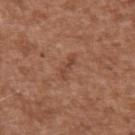Part of a total-body skin-imaging series; this lesion was reviewed on a skin check and was not flagged for biopsy.
The lesion's longest dimension is about 3.5 mm.
The lesion is on the left upper arm.
A male patient, aged 73 to 77.
Automated image analysis of the tile measured an outline eccentricity of about 0.9 (0 = round, 1 = elongated) and a symmetry-axis asymmetry near 0.35. It also reported a border-irregularity index near 4.5/10. And it measured a classifier nevus-likeness of about 0/100.
Captured under white-light illumination.
A region of skin cropped from a whole-body photographic capture, roughly 15 mm wide.